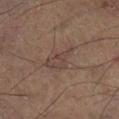Acquisition and patient details: Cropped from a total-body skin-imaging series; the visible field is about 15 mm. Captured under cross-polarized illumination. The subject is a male aged approximately 50. Approximately 4 mm at its widest. The lesion is on the leg.